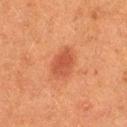<lesion>
  <biopsy_status>not biopsied; imaged during a skin examination</biopsy_status>
  <automated_metrics>
    <color_variation_0_10>1.5</color_variation_0_10>
    <nevus_likeness_0_100>95</nevus_likeness_0_100>
    <lesion_detection_confidence_0_100>100</lesion_detection_confidence_0_100>
  </automated_metrics>
  <image>
    <source>total-body photography crop</source>
    <field_of_view_mm>15</field_of_view_mm>
  </image>
  <site>right thigh</site>
  <lighting>cross-polarized</lighting>
  <lesion_size>
    <long_diameter_mm_approx>3.5</long_diameter_mm_approx>
  </lesion_size>
  <patient>
    <sex>female</sex>
    <age_approx>50</age_approx>
  </patient>
</lesion>